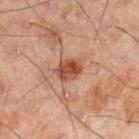{"biopsy_status": "not biopsied; imaged during a skin examination", "site": "right lower leg", "lighting": "cross-polarized", "lesion_size": {"long_diameter_mm_approx": 3.0}, "image": {"source": "total-body photography crop", "field_of_view_mm": 15}, "patient": {"sex": "male", "age_approx": 45}}